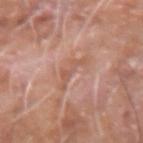Notes:
* workup: imaged on a skin check; not biopsied
* diameter: about 4 mm
* subject: male, aged 73 to 77
* image source: ~15 mm crop, total-body skin-cancer survey
* body site: the right forearm